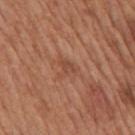body site: the mid back
patient: male, about 65 years old
TBP lesion metrics: a footprint of about 3.5 mm², a shape eccentricity near 0.5, and a shape-asymmetry score of about 0.6 (0 = symmetric); an average lesion color of about L≈48 a*≈24 b*≈32 (CIELAB) and a normalized lesion–skin contrast near 5; a border-irregularity index near 6/10, internal color variation of about 1 on a 0–10 scale, and peripheral color asymmetry of about 0.5; a classifier nevus-likeness of about 0/100 and lesion-presence confidence of about 100/100
image source: ~15 mm tile from a whole-body skin photo
illumination: white-light illumination
diameter: ≈2.5 mm